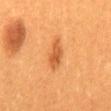Clinical impression:
Imaged during a routine full-body skin examination; the lesion was not biopsied and no histopathology is available.
Clinical summary:
The recorded lesion diameter is about 3.5 mm. From the back. The subject is a female in their 30s. A region of skin cropped from a whole-body photographic capture, roughly 15 mm wide. This is a cross-polarized tile.